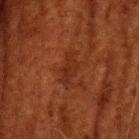{"biopsy_status": "not biopsied; imaged during a skin examination", "lighting": "cross-polarized", "site": "head or neck", "patient": {"sex": "male", "age_approx": 60}, "lesion_size": {"long_diameter_mm_approx": 3.5}, "image": {"source": "total-body photography crop", "field_of_view_mm": 15}}